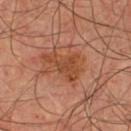Clinical summary:
The lesion is on the chest. A male patient aged approximately 65. A region of skin cropped from a whole-body photographic capture, roughly 15 mm wide.